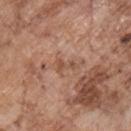biopsy_status: not biopsied; imaged during a skin examination
patient:
  sex: male
  age_approx: 70
lighting: white-light
image:
  source: total-body photography crop
  field_of_view_mm: 15
site: chest